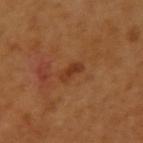{
  "biopsy_status": "not biopsied; imaged during a skin examination",
  "lighting": "cross-polarized",
  "automated_metrics": {
    "cielab_L": 38,
    "cielab_a": 25,
    "cielab_b": 36,
    "vs_skin_contrast_norm": 7.0,
    "nevus_likeness_0_100": 5,
    "lesion_detection_confidence_0_100": 100
  },
  "image": {
    "source": "total-body photography crop",
    "field_of_view_mm": 15
  },
  "patient": {
    "sex": "female",
    "age_approx": 55
  },
  "lesion_size": {
    "long_diameter_mm_approx": 3.0
  },
  "site": "right upper arm"
}